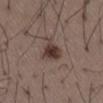{
  "biopsy_status": "not biopsied; imaged during a skin examination",
  "image": {
    "source": "total-body photography crop",
    "field_of_view_mm": 15
  },
  "site": "lower back",
  "patient": {
    "sex": "male",
    "age_approx": 50
  }
}